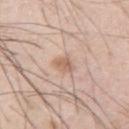Part of a total-body skin-imaging series; this lesion was reviewed on a skin check and was not flagged for biopsy.
An algorithmic analysis of the crop reported a footprint of about 3.5 mm² and an eccentricity of roughly 0.8. The software also gave a within-lesion color-variation index near 1.5/10 and a peripheral color-asymmetry measure near 0.5. It also reported an automated nevus-likeness rating near 40 out of 100 and a lesion-detection confidence of about 100/100.
A male patient roughly 55 years of age.
Cropped from a total-body skin-imaging series; the visible field is about 15 mm.
Longest diameter approximately 2.5 mm.